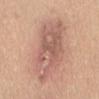follow-up: catalogued during a skin exam; not biopsied | image: 15 mm crop, total-body photography | patient: female, aged approximately 45 | lesion size: ≈11 mm | illumination: white-light | automated lesion analysis: a footprint of about 33 mm² and a shape-asymmetry score of about 0.2 (0 = symmetric); a lesion color around L≈60 a*≈21 b*≈27 in CIELAB, about 10 CIELAB-L* units darker than the surrounding skin, and a normalized lesion–skin contrast near 7; a border-irregularity index near 4/10, a color-variation rating of about 6/10, and a peripheral color-asymmetry measure near 2 | site: the lower back.